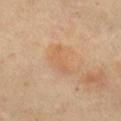The lesion was tiled from a total-body skin photograph and was not biopsied.